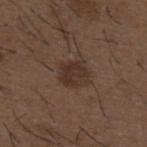The lesion was photographed on a routine skin check and not biopsied; there is no pathology result. A close-up tile cropped from a whole-body skin photograph, about 15 mm across. The recorded lesion diameter is about 3 mm. A male subject aged 48 to 52. The lesion is on the upper back.